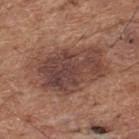No biopsy was performed on this lesion — it was imaged during a full skin examination and was not determined to be concerning.